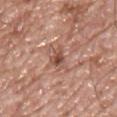Q: Was a biopsy performed?
A: total-body-photography surveillance lesion; no biopsy
Q: How was the tile lit?
A: white-light
Q: What kind of image is this?
A: 15 mm crop, total-body photography
Q: Lesion size?
A: ~3 mm (longest diameter)
Q: Who is the patient?
A: male, in their mid- to late 50s